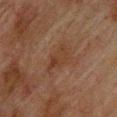Assessment:
Captured during whole-body skin photography for melanoma surveillance; the lesion was not biopsied.
Context:
The tile uses cross-polarized illumination. A male subject, roughly 75 years of age. A close-up tile cropped from a whole-body skin photograph, about 15 mm across. The recorded lesion diameter is about 4 mm. Located on the upper back. An algorithmic analysis of the crop reported a footprint of about 6.5 mm², an outline eccentricity of about 0.8 (0 = round, 1 = elongated), and two-axis asymmetry of about 0.35. And it measured an average lesion color of about L≈31 a*≈17 b*≈25 (CIELAB) and a lesion-to-skin contrast of about 5.5 (normalized; higher = more distinct). The software also gave border irregularity of about 4 on a 0–10 scale, a within-lesion color-variation index near 3/10, and a peripheral color-asymmetry measure near 1. It also reported a nevus-likeness score of about 0/100 and lesion-presence confidence of about 100/100.